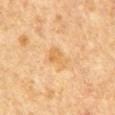This lesion was catalogued during total-body skin photography and was not selected for biopsy. A male patient in their mid- to late 60s. An algorithmic analysis of the crop reported an average lesion color of about L≈61 a*≈19 b*≈41 (CIELAB), roughly 7 lightness units darker than nearby skin, and a lesion-to-skin contrast of about 6 (normalized; higher = more distinct). It also reported border irregularity of about 3.5 on a 0–10 scale, a color-variation rating of about 2.5/10, and radial color variation of about 1. The analysis additionally found an automated nevus-likeness rating near 0 out of 100 and a lesion-detection confidence of about 100/100. The lesion is located on the front of the torso. This is a cross-polarized tile. A close-up tile cropped from a whole-body skin photograph, about 15 mm across. About 3 mm across.